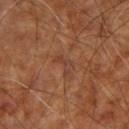Assessment: The lesion was photographed on a routine skin check and not biopsied; there is no pathology result. Acquisition and patient details: A subject roughly 65 years of age. The total-body-photography lesion software estimated an area of roughly 3 mm², an eccentricity of roughly 0.85, and two-axis asymmetry of about 0.65. It also reported a mean CIELAB color near L≈40 a*≈22 b*≈29, a lesion–skin lightness drop of about 5, and a normalized lesion–skin contrast near 5. The software also gave internal color variation of about 0 on a 0–10 scale. And it measured an automated nevus-likeness rating near 0 out of 100. Longest diameter approximately 2.5 mm. A 15 mm close-up extracted from a 3D total-body photography capture. Imaged with cross-polarized lighting. The lesion is on the arm.